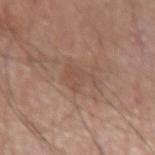Notes:
* notes — total-body-photography surveillance lesion; no biopsy
* location — the right upper arm
* image source — ~15 mm tile from a whole-body skin photo
* patient — male, in their 60s